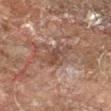follow-up — total-body-photography surveillance lesion; no biopsy | illumination — cross-polarized illumination | TBP lesion metrics — an eccentricity of roughly 0.85; a mean CIELAB color near L≈33 a*≈14 b*≈20 and about 6 CIELAB-L* units darker than the surrounding skin; border irregularity of about 5 on a 0–10 scale; a classifier nevus-likeness of about 0/100 and a lesion-detection confidence of about 70/100 | subject — male, in their 60s | location — the left forearm | imaging modality — ~15 mm crop, total-body skin-cancer survey.